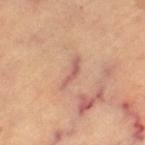{"biopsy_status": "not biopsied; imaged during a skin examination", "lesion_size": {"long_diameter_mm_approx": 4.0}, "patient": {"age_approx": 60}, "image": {"source": "total-body photography crop", "field_of_view_mm": 15}, "site": "leg", "lighting": "cross-polarized", "automated_metrics": {"area_mm2_approx": 3.5, "lesion_detection_confidence_0_100": 65}}